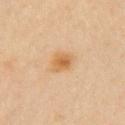| key | value |
|---|---|
| workup | total-body-photography surveillance lesion; no biopsy |
| imaging modality | total-body-photography crop, ~15 mm field of view |
| patient | female, roughly 50 years of age |
| body site | the right upper arm |